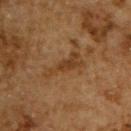The lesion was photographed on a routine skin check and not biopsied; there is no pathology result.
Imaged with cross-polarized lighting.
Automated image analysis of the tile measured an average lesion color of about L≈35 a*≈18 b*≈32 (CIELAB), a lesion–skin lightness drop of about 7, and a normalized border contrast of about 6.5.
On the upper back.
A 15 mm close-up extracted from a 3D total-body photography capture.
The recorded lesion diameter is about 5.5 mm.
A male subject, in their mid-60s.